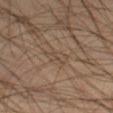Recorded during total-body skin imaging; not selected for excision or biopsy.
An algorithmic analysis of the crop reported an outline eccentricity of about 0.9 (0 = round, 1 = elongated) and a symmetry-axis asymmetry near 0.4. And it measured a lesion color around L≈44 a*≈13 b*≈25 in CIELAB, about 5 CIELAB-L* units darker than the surrounding skin, and a lesion-to-skin contrast of about 4 (normalized; higher = more distinct). The software also gave a classifier nevus-likeness of about 0/100 and a lesion-detection confidence of about 0/100.
This is a cross-polarized tile.
A region of skin cropped from a whole-body photographic capture, roughly 15 mm wide.
A male subject aged 43–47.
On the leg.